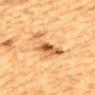Impression:
No biopsy was performed on this lesion — it was imaged during a full skin examination and was not determined to be concerning.
Acquisition and patient details:
Located on the back. A 15 mm close-up extracted from a 3D total-body photography capture. A male patient about 85 years old or older. The tile uses cross-polarized illumination. Automated image analysis of the tile measured a mean CIELAB color near L≈55 a*≈21 b*≈39 and roughly 12 lightness units darker than nearby skin. And it measured border irregularity of about 7 on a 0–10 scale and radial color variation of about 2.